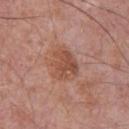Assessment:
This lesion was catalogued during total-body skin photography and was not selected for biopsy.
Acquisition and patient details:
Longest diameter approximately 4 mm. From the chest. A 15 mm crop from a total-body photograph taken for skin-cancer surveillance. The lesion-visualizer software estimated a footprint of about 11 mm², an outline eccentricity of about 0.6 (0 = round, 1 = elongated), and a symmetry-axis asymmetry near 0.25. The patient is a male aged around 75. The tile uses white-light illumination.